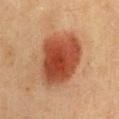Case summary:
• workup — catalogued during a skin exam; not biopsied
• imaging modality — ~15 mm crop, total-body skin-cancer survey
• TBP lesion metrics — a lesion color around L≈41 a*≈26 b*≈31 in CIELAB
• lesion size — about 7 mm
• patient — female, roughly 40 years of age
• site — the front of the torso